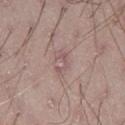subject: male, approximately 20 years of age; lesion size: ≈2.5 mm; imaging modality: 15 mm crop, total-body photography; anatomic site: the leg.